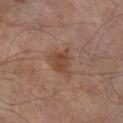Impression: Imaged during a routine full-body skin examination; the lesion was not biopsied and no histopathology is available. Acquisition and patient details: A 15 mm crop from a total-body photograph taken for skin-cancer surveillance. On the right lower leg. The subject is a male aged approximately 65. Automated tile analysis of the lesion measured a lesion color around L≈42 a*≈19 b*≈27 in CIELAB, about 8 CIELAB-L* units darker than the surrounding skin, and a normalized border contrast of about 7. The analysis additionally found a nevus-likeness score of about 50/100 and lesion-presence confidence of about 100/100.